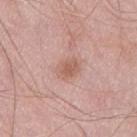Notes:
• notes: total-body-photography surveillance lesion; no biopsy
• imaging modality: ~15 mm tile from a whole-body skin photo
• subject: male, aged approximately 55
• size: ~3 mm (longest diameter)
• site: the left thigh